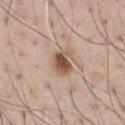Recorded during total-body skin imaging; not selected for excision or biopsy. On the chest. The tile uses white-light illumination. Measured at roughly 3 mm in maximum diameter. A lesion tile, about 15 mm wide, cut from a 3D total-body photograph. A male patient approximately 40 years of age.